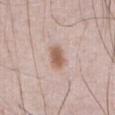{
  "site": "abdomen",
  "image": {
    "source": "total-body photography crop",
    "field_of_view_mm": 15
  },
  "patient": {
    "sex": "male",
    "age_approx": 60
  }
}